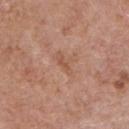A male patient aged 58 to 62.
The lesion-visualizer software estimated a lesion area of about 2 mm² and a shape-asymmetry score of about 0.45 (0 = symmetric). The software also gave an average lesion color of about L≈53 a*≈22 b*≈31 (CIELAB), roughly 7 lightness units darker than nearby skin, and a normalized border contrast of about 5. The analysis additionally found border irregularity of about 5 on a 0–10 scale, internal color variation of about 0 on a 0–10 scale, and peripheral color asymmetry of about 0. It also reported a classifier nevus-likeness of about 0/100 and a lesion-detection confidence of about 100/100.
Cropped from a total-body skin-imaging series; the visible field is about 15 mm.
Located on the chest.